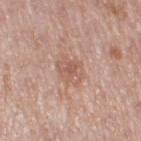This lesion was catalogued during total-body skin photography and was not selected for biopsy.
A female subject, aged 48 to 52.
About 4 mm across.
Imaged with white-light lighting.
A 15 mm close-up tile from a total-body photography series done for melanoma screening.
An algorithmic analysis of the crop reported a footprint of about 7.5 mm², a shape eccentricity near 0.7, and a symmetry-axis asymmetry near 0.2. It also reported a mean CIELAB color near L≈58 a*≈21 b*≈27 and roughly 8 lightness units darker than nearby skin. The software also gave a peripheral color-asymmetry measure near 1.
The lesion is on the right thigh.